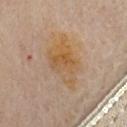Captured during whole-body skin photography for melanoma surveillance; the lesion was not biopsied. The subject is a female about 60 years old. Captured under cross-polarized illumination. Located on the chest. A 15 mm crop from a total-body photograph taken for skin-cancer surveillance.